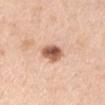Part of a total-body skin-imaging series; this lesion was reviewed on a skin check and was not flagged for biopsy. A male patient, roughly 50 years of age. The lesion is on the right upper arm. This image is a 15 mm lesion crop taken from a total-body photograph.